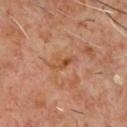Case summary:
* follow-up — imaged on a skin check; not biopsied
* lighting — cross-polarized
* anatomic site — the chest
* lesion size — about 3 mm
* patient — male, approximately 60 years of age
* acquisition — ~15 mm crop, total-body skin-cancer survey